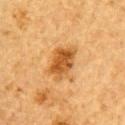Impression: The lesion was photographed on a routine skin check and not biopsied; there is no pathology result. Clinical summary: A close-up tile cropped from a whole-body skin photograph, about 15 mm across. The total-body-photography lesion software estimated a symmetry-axis asymmetry near 0.25. A male patient aged 83 or over. The lesion is on the right upper arm. Captured under cross-polarized illumination. Approximately 4.5 mm at its widest.